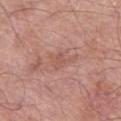Recorded during total-body skin imaging; not selected for excision or biopsy. From the left thigh. An algorithmic analysis of the crop reported a border-irregularity index near 6/10. The lesion's longest dimension is about 3.5 mm. A roughly 15 mm field-of-view crop from a total-body skin photograph. The patient is a male aged around 70.